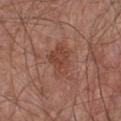biopsy status = no biopsy performed (imaged during a skin exam) | automated lesion analysis = an average lesion color of about L≈44 a*≈23 b*≈28 (CIELAB) | image = total-body-photography crop, ~15 mm field of view | anatomic site = the chest | patient = male, aged around 65.